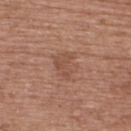Assessment: No biopsy was performed on this lesion — it was imaged during a full skin examination and was not determined to be concerning. Acquisition and patient details: A 15 mm close-up tile from a total-body photography series done for melanoma screening. The patient is a female aged 38–42. The lesion is located on the upper back. The tile uses white-light illumination. Automated tile analysis of the lesion measured an area of roughly 7 mm² and a shape eccentricity near 0.6.